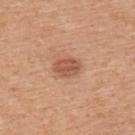follow-up — catalogued during a skin exam; not biopsied
diameter — ≈3 mm
body site — the upper back
lighting — white-light illumination
patient — male, aged 48 to 52
acquisition — 15 mm crop, total-body photography